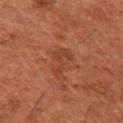No biopsy was performed on this lesion — it was imaged during a full skin examination and was not determined to be concerning.
The lesion is on the left upper arm.
The subject is a male roughly 50 years of age.
The lesion-visualizer software estimated an average lesion color of about L≈31 a*≈22 b*≈27 (CIELAB) and roughly 5 lightness units darker than nearby skin. And it measured a border-irregularity rating of about 9/10, a within-lesion color-variation index near 0.5/10, and radial color variation of about 0. The analysis additionally found an automated nevus-likeness rating near 0 out of 100.
A 15 mm close-up tile from a total-body photography series done for melanoma screening.
Imaged with cross-polarized lighting.
Measured at roughly 4.5 mm in maximum diameter.